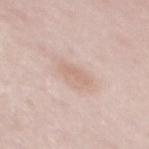Assessment:
Captured during whole-body skin photography for melanoma surveillance; the lesion was not biopsied.
Acquisition and patient details:
The tile uses white-light illumination. A 15 mm close-up tile from a total-body photography series done for melanoma screening. The lesion is on the mid back. About 3 mm across. An algorithmic analysis of the crop reported an average lesion color of about L≈67 a*≈17 b*≈26 (CIELAB), a lesion–skin lightness drop of about 7, and a normalized border contrast of about 4.5. And it measured a within-lesion color-variation index near 1/10 and peripheral color asymmetry of about 0. The analysis additionally found an automated nevus-likeness rating near 0 out of 100 and a lesion-detection confidence of about 100/100. A female patient aged approximately 40.